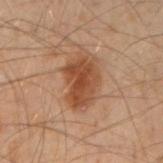Automated tile analysis of the lesion measured an area of roughly 13 mm², a shape eccentricity near 0.65, and a shape-asymmetry score of about 0.25 (0 = symmetric). It also reported an average lesion color of about L≈38 a*≈20 b*≈29 (CIELAB) and a lesion-to-skin contrast of about 8.5 (normalized; higher = more distinct). The analysis additionally found a nevus-likeness score of about 80/100 and lesion-presence confidence of about 100/100.
Cropped from a total-body skin-imaging series; the visible field is about 15 mm.
Located on the left forearm.
The patient is a male approximately 50 years of age.